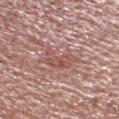Clinical impression:
The lesion was tiled from a total-body skin photograph and was not biopsied.
Image and clinical context:
The lesion's longest dimension is about 3.5 mm. A male subject, aged approximately 65. A region of skin cropped from a whole-body photographic capture, roughly 15 mm wide. The lesion is on the head or neck.